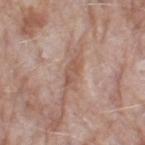biopsy status = imaged on a skin check; not biopsied | image source = total-body-photography crop, ~15 mm field of view | automated metrics = an average lesion color of about L≈54 a*≈20 b*≈27 (CIELAB), a lesion–skin lightness drop of about 8, and a normalized border contrast of about 6 | patient = female, roughly 70 years of age | anatomic site = the left thigh | lesion size = about 3.5 mm | lighting = white-light.